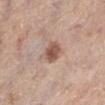Impression:
Recorded during total-body skin imaging; not selected for excision or biopsy.
Acquisition and patient details:
Automated tile analysis of the lesion measured roughly 13 lightness units darker than nearby skin and a normalized lesion–skin contrast near 8.5. The analysis additionally found a nevus-likeness score of about 75/100 and a detector confidence of about 100 out of 100 that the crop contains a lesion. A female subject aged 63 to 67. Cropped from a total-body skin-imaging series; the visible field is about 15 mm. This is a white-light tile. The lesion is on the leg. The recorded lesion diameter is about 3 mm.